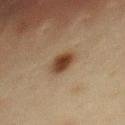Located on the chest. Measured at roughly 3 mm in maximum diameter. A female subject, approximately 40 years of age. Imaged with cross-polarized lighting. A roughly 15 mm field-of-view crop from a total-body skin photograph.